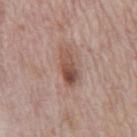Findings:
• follow-up · imaged on a skin check; not biopsied
• anatomic site · the abdomen
• acquisition · total-body-photography crop, ~15 mm field of view
• lighting · white-light illumination
• lesion diameter · about 4.5 mm
• subject · male, approximately 70 years of age